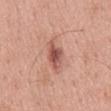  biopsy_status: not biopsied; imaged during a skin examination
  site: mid back
  lighting: white-light
  automated_metrics:
    area_mm2_approx: 6.5
    shape_asymmetry: 0.3
    cielab_L: 54
    cielab_a: 25
    cielab_b: 27
    vs_skin_darker_L: 13.0
    vs_skin_contrast_norm: 8.5
    peripheral_color_asymmetry: 1.5
    nevus_likeness_0_100: 80
    lesion_detection_confidence_0_100: 100
  image:
    source: total-body photography crop
    field_of_view_mm: 15
  patient:
    sex: male
    age_approx: 60